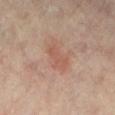| feature | finding |
|---|---|
| workup | no biopsy performed (imaged during a skin exam) |
| lesion diameter | about 3 mm |
| image-analysis metrics | an area of roughly 4.5 mm² and a shape eccentricity near 0.85; peripheral color asymmetry of about 0.5; a classifier nevus-likeness of about 40/100 |
| lighting | cross-polarized illumination |
| image | ~15 mm crop, total-body skin-cancer survey |
| anatomic site | the leg |
| patient | female, aged around 65 |